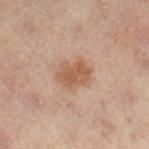Impression:
This lesion was catalogued during total-body skin photography and was not selected for biopsy.
Background:
A region of skin cropped from a whole-body photographic capture, roughly 15 mm wide. A female patient about 55 years old. The lesion is located on the leg. The tile uses cross-polarized illumination. The lesion-visualizer software estimated a lesion area of about 8 mm² and a shape-asymmetry score of about 0.2 (0 = symmetric). The analysis additionally found a mean CIELAB color near L≈46 a*≈17 b*≈27 and about 8 CIELAB-L* units darker than the surrounding skin. It also reported border irregularity of about 2 on a 0–10 scale and internal color variation of about 2.5 on a 0–10 scale. The analysis additionally found an automated nevus-likeness rating near 65 out of 100. About 4 mm across.